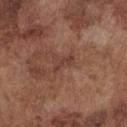Assessment: Part of a total-body skin-imaging series; this lesion was reviewed on a skin check and was not flagged for biopsy. Context: The lesion is on the front of the torso. The tile uses white-light illumination. An algorithmic analysis of the crop reported a lesion-detection confidence of about 100/100. The patient is a male aged 73–77. A 15 mm close-up extracted from a 3D total-body photography capture. The lesion's longest dimension is about 3 mm.